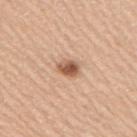| key | value |
|---|---|
| follow-up | imaged on a skin check; not biopsied |
| body site | the right upper arm |
| automated metrics | a shape eccentricity near 0.6 and a shape-asymmetry score of about 0.25 (0 = symmetric); a lesion–skin lightness drop of about 15 and a normalized lesion–skin contrast near 9.5; a border-irregularity rating of about 2/10, a within-lesion color-variation index near 4.5/10, and radial color variation of about 1.5 |
| acquisition | ~15 mm crop, total-body skin-cancer survey |
| lighting | white-light illumination |
| subject | male, roughly 40 years of age |
| size | ~3 mm (longest diameter) |